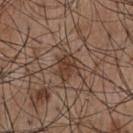Assessment:
This lesion was catalogued during total-body skin photography and was not selected for biopsy.
Background:
On the chest. The tile uses white-light illumination. A 15 mm close-up extracted from a 3D total-body photography capture. The patient is a male aged 53–57. The total-body-photography lesion software estimated a lesion area of about 5.5 mm², a shape eccentricity near 0.75, and two-axis asymmetry of about 0.25. The analysis additionally found a lesion color around L≈38 a*≈18 b*≈26 in CIELAB and a normalized lesion–skin contrast near 8. The analysis additionally found a border-irregularity rating of about 2.5/10, a color-variation rating of about 2.5/10, and radial color variation of about 1.